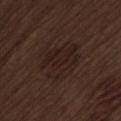{"biopsy_status": "not biopsied; imaged during a skin examination", "site": "lower back", "lesion_size": {"long_diameter_mm_approx": 6.0}, "patient": {"sex": "male", "age_approx": 70}, "image": {"source": "total-body photography crop", "field_of_view_mm": 15}, "automated_metrics": {"area_mm2_approx": 18.0, "eccentricity": 0.6, "shape_asymmetry": 0.25, "border_irregularity_0_10": 3.5, "color_variation_0_10": 2.5}}